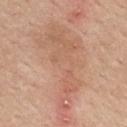Recorded during total-body skin imaging; not selected for excision or biopsy. A region of skin cropped from a whole-body photographic capture, roughly 15 mm wide. A female patient, approximately 65 years of age. Measured at roughly 11.5 mm in maximum diameter. The lesion is on the mid back. Captured under white-light illumination.